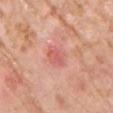- notes — total-body-photography surveillance lesion; no biopsy
- acquisition — 15 mm crop, total-body photography
- patient — female, aged approximately 75
- location — the chest
- tile lighting — white-light